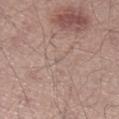Impression:
This lesion was catalogued during total-body skin photography and was not selected for biopsy.
Clinical summary:
The lesion-visualizer software estimated a footprint of about 1 mm², an outline eccentricity of about 0.8 (0 = round, 1 = elongated), and two-axis asymmetry of about 0.45. The analysis additionally found a mean CIELAB color near L≈57 a*≈14 b*≈22 and a normalized border contrast of about 3. The software also gave a border-irregularity index near 3.5/10, internal color variation of about 0 on a 0–10 scale, and a peripheral color-asymmetry measure near 0. And it measured lesion-presence confidence of about 0/100. On the leg. A 15 mm crop from a total-body photograph taken for skin-cancer surveillance. Captured under white-light illumination. Approximately 1 mm at its widest. The subject is a male approximately 40 years of age.